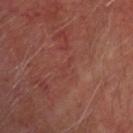Q: Was this lesion biopsied?
A: total-body-photography surveillance lesion; no biopsy
Q: Who is the patient?
A: male, aged approximately 60
Q: Where on the body is the lesion?
A: the head or neck
Q: What is the lesion's diameter?
A: ~2.5 mm (longest diameter)
Q: What is the imaging modality?
A: total-body-photography crop, ~15 mm field of view